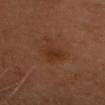lesion size=≈4 mm
imaging modality=total-body-photography crop, ~15 mm field of view
subject=male, aged 53 to 57
lighting=cross-polarized
site=the head or neck